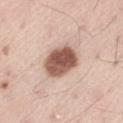Q: Was this lesion biopsied?
A: no biopsy performed (imaged during a skin exam)
Q: How was this image acquired?
A: ~15 mm tile from a whole-body skin photo
Q: What is the anatomic site?
A: the left thigh
Q: Who is the patient?
A: male, aged around 55